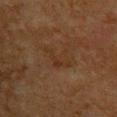biopsy status = total-body-photography surveillance lesion; no biopsy | image = ~15 mm tile from a whole-body skin photo | subject = male, roughly 65 years of age | lesion size = about 3.5 mm | body site = the back.